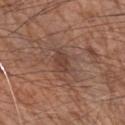No biopsy was performed on this lesion — it was imaged during a full skin examination and was not determined to be concerning. A male patient, aged around 70. A 15 mm close-up tile from a total-body photography series done for melanoma screening. From the left forearm.